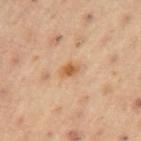Imaged with cross-polarized lighting.
Located on the arm.
A 15 mm close-up tile from a total-body photography series done for melanoma screening.
The patient is a male aged approximately 55.
Measured at roughly 2.5 mm in maximum diameter.